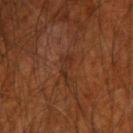| field | value |
|---|---|
| biopsy status | imaged on a skin check; not biopsied |
| image | ~15 mm tile from a whole-body skin photo |
| patient | male, aged 68 to 72 |
| site | the right upper arm |